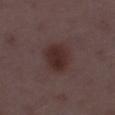Assessment: The lesion was photographed on a routine skin check and not biopsied; there is no pathology result. Acquisition and patient details: A region of skin cropped from a whole-body photographic capture, roughly 15 mm wide. An algorithmic analysis of the crop reported an eccentricity of roughly 0.45. The software also gave a lesion–skin lightness drop of about 8 and a normalized border contrast of about 9. It also reported a lesion-detection confidence of about 100/100. Captured under white-light illumination. The subject is a female roughly 35 years of age. The lesion is located on the left thigh. Measured at roughly 3.5 mm in maximum diameter.